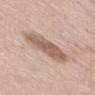Image and clinical context:
Longest diameter approximately 6.5 mm. From the mid back. A region of skin cropped from a whole-body photographic capture, roughly 15 mm wide. A male subject in their 80s. The total-body-photography lesion software estimated a footprint of about 13 mm² and a shape-asymmetry score of about 0.2 (0 = symmetric). It also reported border irregularity of about 3 on a 0–10 scale, a color-variation rating of about 3/10, and a peripheral color-asymmetry measure near 1. The analysis additionally found a classifier nevus-likeness of about 15/100.